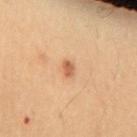The lesion was photographed on a routine skin check and not biopsied; there is no pathology result. On the chest. A female subject, aged 38–42. A region of skin cropped from a whole-body photographic capture, roughly 15 mm wide.